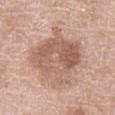Clinical impression:
No biopsy was performed on this lesion — it was imaged during a full skin examination and was not determined to be concerning.
Background:
A female subject, in their 60s. Cropped from a whole-body photographic skin survey; the tile spans about 15 mm. This is a white-light tile. The lesion is located on the right thigh. About 7 mm across. The lesion-visualizer software estimated a within-lesion color-variation index near 6/10 and a peripheral color-asymmetry measure near 2.5. The analysis additionally found a classifier nevus-likeness of about 10/100.